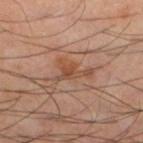Impression: Imaged during a routine full-body skin examination; the lesion was not biopsied and no histopathology is available. Acquisition and patient details: A 15 mm close-up extracted from a 3D total-body photography capture. A male patient aged 38–42. The lesion is located on the leg. The lesion's longest dimension is about 4 mm. The tile uses cross-polarized illumination.